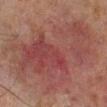  biopsy_status: not biopsied; imaged during a skin examination
  lighting: cross-polarized
  patient:
    sex: male
    age_approx: 65
  automated_metrics:
    lesion_detection_confidence_0_100: 100
  image:
    source: total-body photography crop
    field_of_view_mm: 15
  site: left lower leg